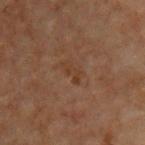Q: Was this lesion biopsied?
A: no biopsy performed (imaged during a skin exam)
Q: Lesion location?
A: the front of the torso
Q: What is the imaging modality?
A: 15 mm crop, total-body photography
Q: Patient demographics?
A: male, about 70 years old